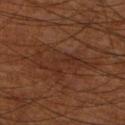Clinical impression:
Recorded during total-body skin imaging; not selected for excision or biopsy.
Background:
A male subject aged approximately 70. The lesion is on the right thigh. This image is a 15 mm lesion crop taken from a total-body photograph.